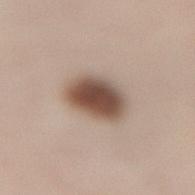biopsy status: total-body-photography surveillance lesion; no biopsy
automated lesion analysis: a symmetry-axis asymmetry near 0.1; a lesion color around L≈50 a*≈16 b*≈25 in CIELAB, roughly 18 lightness units darker than nearby skin, and a normalized border contrast of about 12; a border-irregularity rating of about 1.5/10, a color-variation rating of about 5.5/10, and a peripheral color-asymmetry measure near 1.5
lesion diameter: ≈5 mm
body site: the back
image: total-body-photography crop, ~15 mm field of view
illumination: white-light illumination
subject: female, aged around 50